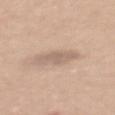Notes:
* follow-up: imaged on a skin check; not biopsied
* imaging modality: total-body-photography crop, ~15 mm field of view
* lighting: white-light
* image-analysis metrics: an area of roughly 5.5 mm² and two-axis asymmetry of about 0.25; border irregularity of about 4 on a 0–10 scale and peripheral color asymmetry of about 0.5
* location: the mid back
* diameter: ~4 mm (longest diameter)
* subject: female, in their mid-50s